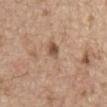Impression:
The lesion was tiled from a total-body skin photograph and was not biopsied.
Acquisition and patient details:
Cropped from a whole-body photographic skin survey; the tile spans about 15 mm. The lesion is located on the abdomen. Imaged with white-light lighting. Approximately 5 mm at its widest. A male patient aged approximately 70.